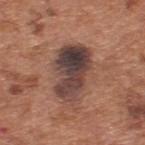workup — total-body-photography surveillance lesion; no biopsy
anatomic site — the upper back
image-analysis metrics — a border-irregularity index near 3.5/10 and a color-variation rating of about 9.5/10; an automated nevus-likeness rating near 0 out of 100 and a lesion-detection confidence of about 100/100
subject — male, roughly 65 years of age
acquisition — ~15 mm crop, total-body skin-cancer survey
tile lighting — white-light illumination